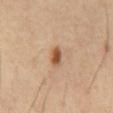Acquisition and patient details: Imaged with cross-polarized lighting. The subject is a male aged approximately 55. The recorded lesion diameter is about 3 mm. Located on the abdomen. Automated image analysis of the tile measured a footprint of about 4 mm², an eccentricity of roughly 0.8, and a symmetry-axis asymmetry near 0.15. The software also gave an average lesion color of about L≈52 a*≈21 b*≈33 (CIELAB) and a normalized lesion–skin contrast near 9. A region of skin cropped from a whole-body photographic capture, roughly 15 mm wide.